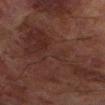Recorded during total-body skin imaging; not selected for excision or biopsy. The lesion is located on the left lower leg. This image is a 15 mm lesion crop taken from a total-body photograph. The patient is a male about 70 years old.